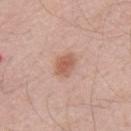This lesion was catalogued during total-body skin photography and was not selected for biopsy. Automated tile analysis of the lesion measured an average lesion color of about L≈58 a*≈22 b*≈29 (CIELAB) and a lesion–skin lightness drop of about 10. And it measured a border-irregularity index near 2.5/10, a within-lesion color-variation index near 2.5/10, and radial color variation of about 1. This is a white-light tile. Approximately 3 mm at its widest. A region of skin cropped from a whole-body photographic capture, roughly 15 mm wide. A male subject approximately 55 years of age. The lesion is located on the back.